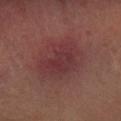| field | value |
|---|---|
| notes | no biopsy performed (imaged during a skin exam) |
| imaging modality | total-body-photography crop, ~15 mm field of view |
| subject | male, approximately 65 years of age |
| diameter | ≈5.5 mm |
| anatomic site | the left forearm |
| lighting | cross-polarized |